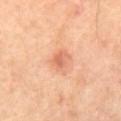follow-up = catalogued during a skin exam; not biopsied
anatomic site = the mid back
lesion size = about 3 mm
automated metrics = an average lesion color of about L≈61 a*≈26 b*≈33 (CIELAB); a border-irregularity index near 2.5/10, internal color variation of about 2.5 on a 0–10 scale, and a peripheral color-asymmetry measure near 1; an automated nevus-likeness rating near 30 out of 100 and a detector confidence of about 100 out of 100 that the crop contains a lesion
lighting = cross-polarized
subject = male, in their mid-50s
imaging modality = 15 mm crop, total-body photography